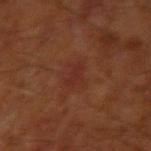Acquisition and patient details:
The lesion-visualizer software estimated a lesion color around L≈28 a*≈25 b*≈26 in CIELAB, a lesion–skin lightness drop of about 4, and a normalized border contrast of about 4.5. The analysis additionally found a border-irregularity index near 4/10, a within-lesion color-variation index near 1/10, and peripheral color asymmetry of about 0.5. It also reported lesion-presence confidence of about 100/100. Imaged with cross-polarized lighting. From the arm. A region of skin cropped from a whole-body photographic capture, roughly 15 mm wide. The lesion's longest dimension is about 2.5 mm. The subject is a male roughly 65 years of age.